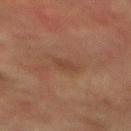The lesion was photographed on a routine skin check and not biopsied; there is no pathology result. The subject is a male about 75 years old. The lesion-visualizer software estimated an area of roughly 3 mm² and a shape eccentricity near 0.8. The analysis additionally found a border-irregularity index near 3/10, a color-variation rating of about 1/10, and peripheral color asymmetry of about 0.5. On the mid back. This image is a 15 mm lesion crop taken from a total-body photograph. The lesion's longest dimension is about 2.5 mm.